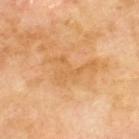Impression:
Imaged during a routine full-body skin examination; the lesion was not biopsied and no histopathology is available.
Acquisition and patient details:
Cropped from a total-body skin-imaging series; the visible field is about 15 mm. On the upper back. Automated image analysis of the tile measured a footprint of about 11 mm², an outline eccentricity of about 0.8 (0 = round, 1 = elongated), and two-axis asymmetry of about 0.65. It also reported a mean CIELAB color near L≈62 a*≈21 b*≈43, about 6 CIELAB-L* units darker than the surrounding skin, and a normalized lesion–skin contrast near 4.5. The software also gave a detector confidence of about 100 out of 100 that the crop contains a lesion. A male patient aged 68–72. About 5.5 mm across.